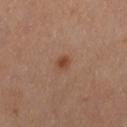Imaged during a routine full-body skin examination; the lesion was not biopsied and no histopathology is available. A female patient in their 60s. The lesion is on the leg. A lesion tile, about 15 mm wide, cut from a 3D total-body photograph.